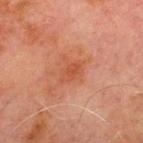notes: catalogued during a skin exam; not biopsied | lighting: cross-polarized | image source: ~15 mm crop, total-body skin-cancer survey | subject: male, aged around 70 | lesion size: ≈3 mm | automated metrics: a lesion area of about 3 mm² and two-axis asymmetry of about 0.3; a lesion color around L≈42 a*≈26 b*≈32 in CIELAB, a lesion–skin lightness drop of about 6, and a normalized border contrast of about 5.5; a classifier nevus-likeness of about 0/100 and lesion-presence confidence of about 100/100 | site: the chest.